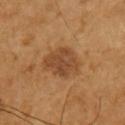Imaged during a routine full-body skin examination; the lesion was not biopsied and no histopathology is available. The patient is a male in their mid-60s. The lesion is located on the left upper arm. The tile uses cross-polarized illumination. A region of skin cropped from a whole-body photographic capture, roughly 15 mm wide.